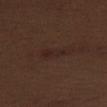Part of a total-body skin-imaging series; this lesion was reviewed on a skin check and was not flagged for biopsy. The lesion's longest dimension is about 4 mm. The total-body-photography lesion software estimated a shape eccentricity near 0.95 and a symmetry-axis asymmetry near 0.3. The analysis additionally found a mean CIELAB color near L≈23 a*≈16 b*≈20 and about 4 CIELAB-L* units darker than the surrounding skin. A 15 mm close-up extracted from a 3D total-body photography capture. The tile uses white-light illumination. The patient is a male roughly 70 years of age. On the left upper arm.